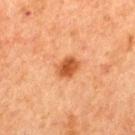Q: Was a biopsy performed?
A: no biopsy performed (imaged during a skin exam)
Q: Who is the patient?
A: male, aged approximately 65
Q: What is the imaging modality?
A: 15 mm crop, total-body photography
Q: Where on the body is the lesion?
A: the mid back